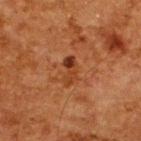Impression: Imaged during a routine full-body skin examination; the lesion was not biopsied and no histopathology is available. Acquisition and patient details: A male subject in their 60s. The recorded lesion diameter is about 3.5 mm. The lesion is on the upper back. A roughly 15 mm field-of-view crop from a total-body skin photograph. This is a cross-polarized tile. The lesion-visualizer software estimated a lesion area of about 4 mm², an outline eccentricity of about 0.9 (0 = round, 1 = elongated), and two-axis asymmetry of about 0.35. And it measured an average lesion color of about L≈29 a*≈22 b*≈30 (CIELAB), roughly 8 lightness units darker than nearby skin, and a normalized border contrast of about 8. It also reported a classifier nevus-likeness of about 5/100 and a lesion-detection confidence of about 100/100.